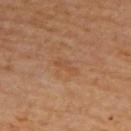Clinical impression:
Part of a total-body skin-imaging series; this lesion was reviewed on a skin check and was not flagged for biopsy.
Image and clinical context:
A 15 mm crop from a total-body photograph taken for skin-cancer surveillance. A female patient, approximately 65 years of age. Captured under cross-polarized illumination. The lesion's longest dimension is about 3 mm. Located on the upper back.